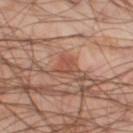No biopsy was performed on this lesion — it was imaged during a full skin examination and was not determined to be concerning.